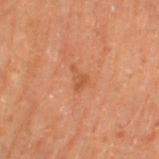Impression: The lesion was tiled from a total-body skin photograph and was not biopsied. Clinical summary: Automated image analysis of the tile measured a lesion color around L≈41 a*≈21 b*≈30 in CIELAB and a normalized lesion–skin contrast near 5. It also reported a border-irregularity rating of about 5.5/10, internal color variation of about 1 on a 0–10 scale, and radial color variation of about 0. A male subject aged around 70. From the right thigh. This is a cross-polarized tile. A close-up tile cropped from a whole-body skin photograph, about 15 mm across. Longest diameter approximately 3.5 mm.